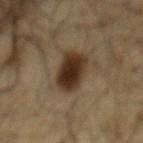Context:
Imaged with cross-polarized lighting. From the back. The lesion-visualizer software estimated a footprint of about 12 mm², an outline eccentricity of about 0.85 (0 = round, 1 = elongated), and a symmetry-axis asymmetry near 0.25. It also reported a mean CIELAB color near L≈29 a*≈13 b*≈26 and roughly 14 lightness units darker than nearby skin. A male patient, aged 63–67. Longest diameter approximately 5.5 mm. Cropped from a whole-body photographic skin survey; the tile spans about 15 mm.